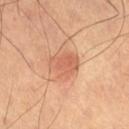No biopsy was performed on this lesion — it was imaged during a full skin examination and was not determined to be concerning.
Located on the abdomen.
Cropped from a whole-body photographic skin survey; the tile spans about 15 mm.
A male patient aged around 70.
Captured under cross-polarized illumination.
The total-body-photography lesion software estimated a lesion area of about 8.5 mm², a shape eccentricity near 0.6, and two-axis asymmetry of about 0.25. The analysis additionally found a border-irregularity rating of about 2.5/10, internal color variation of about 2.5 on a 0–10 scale, and radial color variation of about 1.